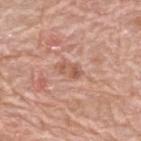{
  "biopsy_status": "not biopsied; imaged during a skin examination",
  "site": "upper back",
  "image": {
    "source": "total-body photography crop",
    "field_of_view_mm": 15
  },
  "patient": {
    "sex": "male",
    "age_approx": 80
  }
}